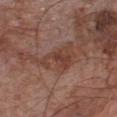Clinical summary:
A lesion tile, about 15 mm wide, cut from a 3D total-body photograph. The lesion's longest dimension is about 5.5 mm. This is a white-light tile. Located on the chest. An algorithmic analysis of the crop reported a border-irregularity index near 8/10, a color-variation rating of about 3/10, and radial color variation of about 1. It also reported a nevus-likeness score of about 0/100 and a lesion-detection confidence of about 95/100. The patient is a male aged around 65.